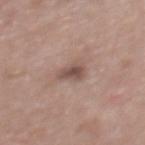Imaged during a routine full-body skin examination; the lesion was not biopsied and no histopathology is available. A close-up tile cropped from a whole-body skin photograph, about 15 mm across. Automated tile analysis of the lesion measured a border-irregularity index near 3/10, a within-lesion color-variation index near 2.5/10, and a peripheral color-asymmetry measure near 1. The lesion's longest dimension is about 3 mm. A female patient, approximately 50 years of age. The tile uses white-light illumination. On the upper back.